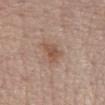Part of a total-body skin-imaging series; this lesion was reviewed on a skin check and was not flagged for biopsy. A close-up tile cropped from a whole-body skin photograph, about 15 mm across. This is a white-light tile. The lesion's longest dimension is about 3.5 mm. A female subject aged 68–72. On the abdomen.